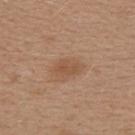Image and clinical context:
Located on the back. The subject is a female aged 38 to 42. A lesion tile, about 15 mm wide, cut from a 3D total-body photograph.